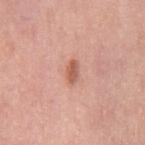Q: Is there a histopathology result?
A: catalogued during a skin exam; not biopsied
Q: What is the lesion's diameter?
A: about 2.5 mm
Q: What is the imaging modality?
A: total-body-photography crop, ~15 mm field of view
Q: What did automated image analysis measure?
A: an automated nevus-likeness rating near 95 out of 100
Q: Lesion location?
A: the right thigh
Q: Who is the patient?
A: female, in their 50s